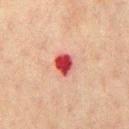| field | value |
|---|---|
| workup | imaged on a skin check; not biopsied |
| image source | ~15 mm crop, total-body skin-cancer survey |
| size | about 3 mm |
| body site | the front of the torso |
| subject | male, approximately 60 years of age |